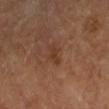* biopsy status: total-body-photography surveillance lesion; no biopsy
* patient: in their 60s
* lesion diameter: ~2.5 mm (longest diameter)
* acquisition: 15 mm crop, total-body photography
* anatomic site: the left forearm
* image-analysis metrics: a lesion color around L≈36 a*≈21 b*≈30 in CIELAB